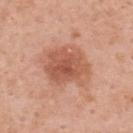Q: Is there a histopathology result?
A: catalogued during a skin exam; not biopsied
Q: Automated lesion metrics?
A: a lesion area of about 21 mm²; a lesion color around L≈57 a*≈25 b*≈32 in CIELAB, a lesion–skin lightness drop of about 11, and a normalized border contrast of about 7
Q: What is the anatomic site?
A: the upper back
Q: How was the tile lit?
A: white-light illumination
Q: What is the imaging modality?
A: ~15 mm crop, total-body skin-cancer survey
Q: Patient demographics?
A: female, aged 48–52
Q: Lesion size?
A: about 5.5 mm